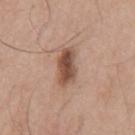{"biopsy_status": "not biopsied; imaged during a skin examination", "site": "mid back", "patient": {"sex": "male", "age_approx": 75}, "lesion_size": {"long_diameter_mm_approx": 4.5}, "lighting": "white-light", "image": {"source": "total-body photography crop", "field_of_view_mm": 15}}